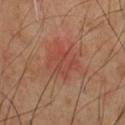• biopsy status: no biopsy performed (imaged during a skin exam)
• body site: the chest
• subject: male, aged around 60
• size: about 4 mm
• image source: ~15 mm crop, total-body skin-cancer survey
• tile lighting: cross-polarized illumination